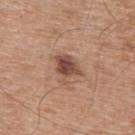Assessment:
Imaged during a routine full-body skin examination; the lesion was not biopsied and no histopathology is available.
Clinical summary:
This is a white-light tile. Measured at roughly 3.5 mm in maximum diameter. The lesion is located on the upper back. A 15 mm close-up tile from a total-body photography series done for melanoma screening. A male patient, aged around 65. The lesion-visualizer software estimated a lesion area of about 7 mm², a shape eccentricity near 0.6, and a shape-asymmetry score of about 0.35 (0 = symmetric). It also reported a lesion color around L≈48 a*≈21 b*≈28 in CIELAB, a lesion–skin lightness drop of about 13, and a normalized lesion–skin contrast near 9.5. The analysis additionally found border irregularity of about 4 on a 0–10 scale and a peripheral color-asymmetry measure near 2.